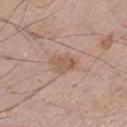The lesion was tiled from a total-body skin photograph and was not biopsied. This is a white-light tile. A male subject, aged 68 to 72. On the right thigh. Automated image analysis of the tile measured a footprint of about 5.5 mm². And it measured a border-irregularity index near 3.5/10. It also reported an automated nevus-likeness rating near 10 out of 100. A roughly 15 mm field-of-view crop from a total-body skin photograph.